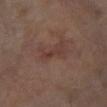{
  "biopsy_status": "not biopsied; imaged during a skin examination",
  "lighting": "cross-polarized",
  "patient": {
    "sex": "male",
    "age_approx": 70
  },
  "site": "left lower leg",
  "image": {
    "source": "total-body photography crop",
    "field_of_view_mm": 15
  },
  "lesion_size": {
    "long_diameter_mm_approx": 3.0
  }
}